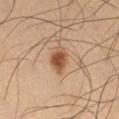This is a cross-polarized tile. Automated tile analysis of the lesion measured an average lesion color of about L≈42 a*≈17 b*≈28 (CIELAB), roughly 11 lightness units darker than nearby skin, and a normalized lesion–skin contrast near 9. It also reported border irregularity of about 2.5 on a 0–10 scale, a color-variation rating of about 4/10, and radial color variation of about 1. It also reported a classifier nevus-likeness of about 90/100 and lesion-presence confidence of about 100/100. The recorded lesion diameter is about 3 mm. A male subject in their mid- to late 60s. A 15 mm crop from a total-body photograph taken for skin-cancer surveillance. The lesion is on the right thigh.